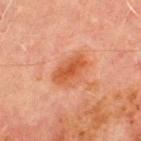Notes:
– diameter — ~4.5 mm (longest diameter)
– location — the chest
– image — total-body-photography crop, ~15 mm field of view
– patient — male, in their 70s